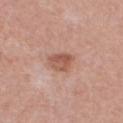Q: Was a biopsy performed?
A: imaged on a skin check; not biopsied
Q: Patient demographics?
A: male, aged 53–57
Q: Lesion location?
A: the chest
Q: What is the imaging modality?
A: ~15 mm crop, total-body skin-cancer survey